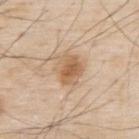Findings:
– notes — total-body-photography surveillance lesion; no biopsy
– image — 15 mm crop, total-body photography
– patient — male, aged approximately 80
– site — the upper back